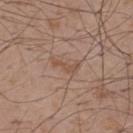Imaged during a routine full-body skin examination; the lesion was not biopsied and no histopathology is available.
A male patient about 50 years old.
The lesion is on the back.
A 15 mm crop from a total-body photograph taken for skin-cancer surveillance.
Measured at roughly 3 mm in maximum diameter.